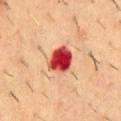Part of a total-body skin-imaging series; this lesion was reviewed on a skin check and was not flagged for biopsy.
Located on the mid back.
About 3.5 mm across.
A male patient, approximately 55 years of age.
A close-up tile cropped from a whole-body skin photograph, about 15 mm across.
Captured under cross-polarized illumination.
An algorithmic analysis of the crop reported a lesion color around L≈42 a*≈40 b*≈32 in CIELAB and about 21 CIELAB-L* units darker than the surrounding skin. And it measured a border-irregularity index near 2/10, a color-variation rating of about 6/10, and a peripheral color-asymmetry measure near 2. It also reported a classifier nevus-likeness of about 0/100 and a detector confidence of about 100 out of 100 that the crop contains a lesion.